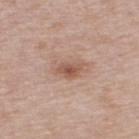– workup: catalogued during a skin exam; not biopsied
– image source: total-body-photography crop, ~15 mm field of view
– automated lesion analysis: an average lesion color of about L≈54 a*≈20 b*≈28 (CIELAB), a lesion–skin lightness drop of about 10, and a lesion-to-skin contrast of about 7 (normalized; higher = more distinct); border irregularity of about 3 on a 0–10 scale and a color-variation rating of about 4.5/10
– lighting: white-light illumination
– lesion diameter: ≈2.5 mm
– site: the upper back
– subject: male, about 55 years old